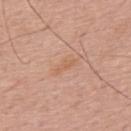Impression: This lesion was catalogued during total-body skin photography and was not selected for biopsy. Background: Automated image analysis of the tile measured a border-irregularity index near 5/10, a within-lesion color-variation index near 0.5/10, and peripheral color asymmetry of about 0. A male patient, approximately 55 years of age. Captured under white-light illumination. This image is a 15 mm lesion crop taken from a total-body photograph. Longest diameter approximately 3 mm.